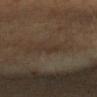Captured during whole-body skin photography for melanoma surveillance; the lesion was not biopsied. A female patient, roughly 45 years of age. The tile uses cross-polarized illumination. The lesion is on the right forearm. A 15 mm close-up extracted from a 3D total-body photography capture. Longest diameter approximately 3.5 mm.